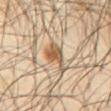Q: Was a biopsy performed?
A: total-body-photography surveillance lesion; no biopsy
Q: Where on the body is the lesion?
A: the abdomen
Q: How large is the lesion?
A: about 4 mm
Q: What are the patient's age and sex?
A: male, aged approximately 65
Q: How was this image acquired?
A: ~15 mm tile from a whole-body skin photo